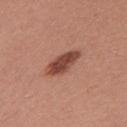Captured during whole-body skin photography for melanoma surveillance; the lesion was not biopsied. This is a white-light tile. From the left upper arm. An algorithmic analysis of the crop reported a lesion area of about 7.5 mm². The software also gave a classifier nevus-likeness of about 80/100 and a detector confidence of about 100 out of 100 that the crop contains a lesion. Measured at roughly 4.5 mm in maximum diameter. A female subject, aged around 25. A 15 mm close-up tile from a total-body photography series done for melanoma screening.